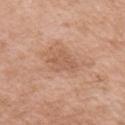Notes:
– workup: no biopsy performed (imaged during a skin exam)
– anatomic site: the right upper arm
– size: ~3.5 mm (longest diameter)
– image source: ~15 mm tile from a whole-body skin photo
– illumination: white-light illumination
– patient: female, in their mid-50s